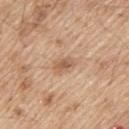– follow-up — no biopsy performed (imaged during a skin exam)
– site — the back
– subject — male, roughly 70 years of age
– size — ~3 mm (longest diameter)
– illumination — white-light
– acquisition — ~15 mm crop, total-body skin-cancer survey
– TBP lesion metrics — a lesion area of about 4 mm² and a shape-asymmetry score of about 0.25 (0 = symmetric); an average lesion color of about L≈59 a*≈19 b*≈33 (CIELAB), about 10 CIELAB-L* units darker than the surrounding skin, and a lesion-to-skin contrast of about 6.5 (normalized; higher = more distinct)